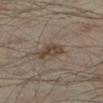This lesion was catalogued during total-body skin photography and was not selected for biopsy.
The patient is a male in their mid- to late 40s.
A lesion tile, about 15 mm wide, cut from a 3D total-body photograph.
On the left lower leg.
This is a cross-polarized tile.
Approximately 4 mm at its widest.